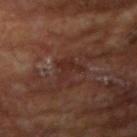Q: Was this lesion biopsied?
A: total-body-photography surveillance lesion; no biopsy
Q: What are the patient's age and sex?
A: male, aged approximately 65
Q: Lesion size?
A: ≈3 mm
Q: Automated lesion metrics?
A: a lesion–skin lightness drop of about 6; a detector confidence of about 80 out of 100 that the crop contains a lesion
Q: What kind of image is this?
A: ~15 mm crop, total-body skin-cancer survey
Q: How was the tile lit?
A: cross-polarized illumination
Q: Where on the body is the lesion?
A: the arm